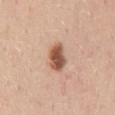| field | value |
|---|---|
| notes | no biopsy performed (imaged during a skin exam) |
| illumination | white-light |
| body site | the mid back |
| subject | female, in their mid-40s |
| acquisition | ~15 mm tile from a whole-body skin photo |
| lesion size | about 4 mm |